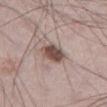Impression:
No biopsy was performed on this lesion — it was imaged during a full skin examination and was not determined to be concerning.
Image and clinical context:
The lesion is on the left thigh. A close-up tile cropped from a whole-body skin photograph, about 15 mm across. The lesion-visualizer software estimated a border-irregularity rating of about 2/10 and peripheral color asymmetry of about 1.5. It also reported a detector confidence of about 100 out of 100 that the crop contains a lesion. Measured at roughly 4 mm in maximum diameter. The tile uses white-light illumination. The patient is a male aged 68–72.